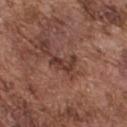The lesion was photographed on a routine skin check and not biopsied; there is no pathology result.
Approximately 3.5 mm at its widest.
A close-up tile cropped from a whole-body skin photograph, about 15 mm across.
The tile uses white-light illumination.
The lesion is located on the upper back.
The patient is a male aged 73 to 77.